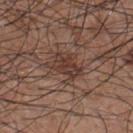Captured during whole-body skin photography for melanoma surveillance; the lesion was not biopsied.